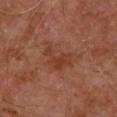follow-up = total-body-photography surveillance lesion; no biopsy | lesion diameter = ≈5 mm | image source = total-body-photography crop, ~15 mm field of view | anatomic site = the upper back | subject = male, approximately 70 years of age.